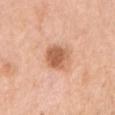• imaging modality — ~15 mm tile from a whole-body skin photo
• subject — female, aged 58 to 62
• lesion size — ≈4 mm
• anatomic site — the right upper arm
• automated lesion analysis — border irregularity of about 2 on a 0–10 scale, a color-variation rating of about 3/10, and a peripheral color-asymmetry measure near 1; a nevus-likeness score of about 80/100 and lesion-presence confidence of about 100/100